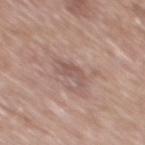Q: Was a biopsy performed?
A: no biopsy performed (imaged during a skin exam)
Q: What lighting was used for the tile?
A: white-light
Q: What is the anatomic site?
A: the mid back
Q: What is the imaging modality?
A: 15 mm crop, total-body photography
Q: What are the patient's age and sex?
A: male, approximately 70 years of age
Q: How large is the lesion?
A: about 3 mm